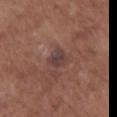– biopsy status: catalogued during a skin exam; not biopsied
– location: the upper back
– size: ≈2.5 mm
– patient: female, in their mid-70s
– automated lesion analysis: an eccentricity of roughly 0.5 and a shape-asymmetry score of about 0.2 (0 = symmetric); an average lesion color of about L≈39 a*≈18 b*≈19 (CIELAB), a lesion–skin lightness drop of about 8, and a normalized lesion–skin contrast near 8
– imaging modality: ~15 mm crop, total-body skin-cancer survey